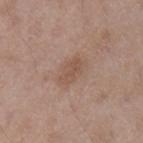| key | value |
|---|---|
| follow-up | imaged on a skin check; not biopsied |
| subject | male, aged approximately 50 |
| image source | 15 mm crop, total-body photography |
| lighting | white-light |
| lesion size | ~3.5 mm (longest diameter) |
| site | the left upper arm |